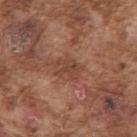Q: Was this lesion biopsied?
A: total-body-photography surveillance lesion; no biopsy
Q: Lesion location?
A: the right upper arm
Q: What kind of image is this?
A: ~15 mm tile from a whole-body skin photo
Q: What are the patient's age and sex?
A: male, about 75 years old
Q: Illumination type?
A: white-light
Q: What did automated image analysis measure?
A: a footprint of about 5.5 mm² and a shape eccentricity near 0.65; a nevus-likeness score of about 0/100 and a detector confidence of about 100 out of 100 that the crop contains a lesion